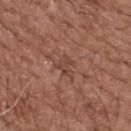notes = no biopsy performed (imaged during a skin exam) | image source = total-body-photography crop, ~15 mm field of view | patient = male, aged around 75 | anatomic site = the upper back.